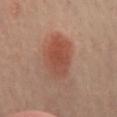Q: Is there a histopathology result?
A: catalogued during a skin exam; not biopsied
Q: What kind of image is this?
A: 15 mm crop, total-body photography
Q: What is the lesion's diameter?
A: ≈6 mm
Q: How was the tile lit?
A: cross-polarized illumination
Q: What did automated image analysis measure?
A: a lesion area of about 16 mm², an eccentricity of roughly 0.8, and a shape-asymmetry score of about 0.15 (0 = symmetric); a lesion color around L≈50 a*≈25 b*≈30 in CIELAB, a lesion–skin lightness drop of about 9, and a lesion-to-skin contrast of about 7.5 (normalized; higher = more distinct); an automated nevus-likeness rating near 100 out of 100 and lesion-presence confidence of about 100/100
Q: Where on the body is the lesion?
A: the abdomen